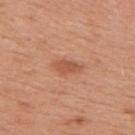<lesion>
  <biopsy_status>not biopsied; imaged during a skin examination</biopsy_status>
  <image>
    <source>total-body photography crop</source>
    <field_of_view_mm>15</field_of_view_mm>
  </image>
  <lighting>white-light</lighting>
  <patient>
    <sex>female</sex>
    <age_approx>50</age_approx>
  </patient>
  <lesion_size>
    <long_diameter_mm_approx>3.0</long_diameter_mm_approx>
  </lesion_size>
  <site>right upper arm</site>
</lesion>